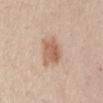Impression: Imaged during a routine full-body skin examination; the lesion was not biopsied and no histopathology is available. Context: The subject is a female aged around 45. Cropped from a whole-body photographic skin survey; the tile spans about 15 mm. The lesion is on the front of the torso.